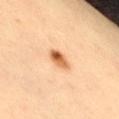biopsy_status: not biopsied; imaged during a skin examination
lesion_size:
  long_diameter_mm_approx: 3.0
site: right thigh
image:
  source: total-body photography crop
  field_of_view_mm: 15
lighting: cross-polarized
patient:
  sex: male
  age_approx: 40